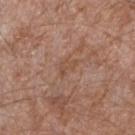Part of a total-body skin-imaging series; this lesion was reviewed on a skin check and was not flagged for biopsy.
This is a white-light tile.
A male subject, aged 53 to 57.
A region of skin cropped from a whole-body photographic capture, roughly 15 mm wide.
Located on the arm.
Measured at roughly 2.5 mm in maximum diameter.